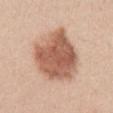- biopsy status — no biopsy performed (imaged during a skin exam)
- subject — female, roughly 40 years of age
- image — 15 mm crop, total-body photography
- body site — the left upper arm